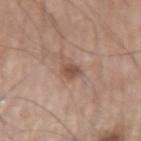Q: Is there a histopathology result?
A: imaged on a skin check; not biopsied
Q: Lesion location?
A: the left upper arm
Q: What lighting was used for the tile?
A: white-light
Q: How was this image acquired?
A: 15 mm crop, total-body photography
Q: Patient demographics?
A: male, approximately 75 years of age